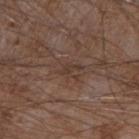biopsy_status: not biopsied; imaged during a skin examination
automated_metrics:
  area_mm2_approx: 2.0
  eccentricity: 0.95
  shape_asymmetry: 0.5
  cielab_L: 36
  cielab_a: 16
  cielab_b: 23
  vs_skin_darker_L: 6.0
  vs_skin_contrast_norm: 5.5
  nevus_likeness_0_100: 0
site: right forearm
lesion_size:
  long_diameter_mm_approx: 2.5
image:
  source: total-body photography crop
  field_of_view_mm: 15
lighting: white-light
patient:
  sex: male
  age_approx: 75